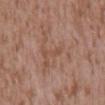Recorded during total-body skin imaging; not selected for excision or biopsy. The tile uses white-light illumination. This image is a 15 mm lesion crop taken from a total-body photograph. Measured at roughly 3 mm in maximum diameter. On the back. The patient is a male about 45 years old. The total-body-photography lesion software estimated a footprint of about 2.5 mm² and a shape eccentricity near 0.95. The software also gave a mean CIELAB color near L≈50 a*≈20 b*≈29 and about 6 CIELAB-L* units darker than the surrounding skin. The analysis additionally found internal color variation of about 0 on a 0–10 scale and a peripheral color-asymmetry measure near 0. The software also gave a nevus-likeness score of about 0/100.